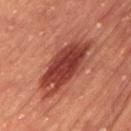workup=imaged on a skin check; not biopsied | image source=total-body-photography crop, ~15 mm field of view | lighting=cross-polarized illumination | body site=the left thigh | image-analysis metrics=a lesion area of about 24 mm², a shape eccentricity near 0.9, and a symmetry-axis asymmetry near 0.2; border irregularity of about 3.5 on a 0–10 scale, internal color variation of about 5.5 on a 0–10 scale, and radial color variation of about 2 | patient=female, aged approximately 60.